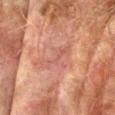A male subject in their mid- to late 70s. Captured under cross-polarized illumination. A 15 mm close-up tile from a total-body photography series done for melanoma screening. From the left forearm.